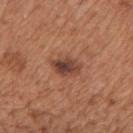<record>
<biopsy_status>not biopsied; imaged during a skin examination</biopsy_status>
</record>